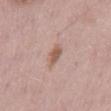| field | value |
|---|---|
| follow-up | catalogued during a skin exam; not biopsied |
| diameter | ~3 mm (longest diameter) |
| site | the back |
| illumination | white-light illumination |
| patient | male, approximately 55 years of age |
| imaging modality | total-body-photography crop, ~15 mm field of view |